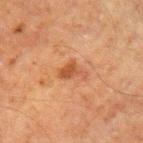| field | value |
|---|---|
| tile lighting | cross-polarized illumination |
| TBP lesion metrics | a lesion area of about 3.5 mm², an outline eccentricity of about 0.8 (0 = round, 1 = elongated), and two-axis asymmetry of about 0.45; a mean CIELAB color near L≈41 a*≈22 b*≈32; a nevus-likeness score of about 30/100 and a lesion-detection confidence of about 100/100 |
| anatomic site | the left upper arm |
| acquisition | ~15 mm crop, total-body skin-cancer survey |
| patient | male, approximately 60 years of age |
| size | about 3 mm |